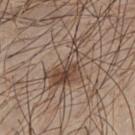– biopsy status · catalogued during a skin exam; not biopsied
– image source · ~15 mm tile from a whole-body skin photo
– patient · male, aged approximately 40
– automated metrics · a lesion color around L≈45 a*≈15 b*≈25 in CIELAB; a nevus-likeness score of about 70/100 and a lesion-detection confidence of about 100/100
– site · the chest
– lesion diameter · ~6.5 mm (longest diameter)